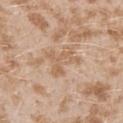No biopsy was performed on this lesion — it was imaged during a full skin examination and was not determined to be concerning. Automated tile analysis of the lesion measured an area of roughly 8 mm², an outline eccentricity of about 0.65 (0 = round, 1 = elongated), and a symmetry-axis asymmetry near 0.65. The analysis additionally found a classifier nevus-likeness of about 0/100. Located on the left upper arm. Measured at roughly 4.5 mm in maximum diameter. Cropped from a whole-body photographic skin survey; the tile spans about 15 mm. A female patient aged around 25. The tile uses white-light illumination.